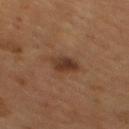{
  "biopsy_status": "not biopsied; imaged during a skin examination",
  "patient": {
    "sex": "male",
    "age_approx": 55
  },
  "site": "back",
  "image": {
    "source": "total-body photography crop",
    "field_of_view_mm": 15
  }
}